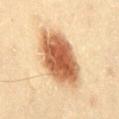Image and clinical context:
A 15 mm close-up extracted from a 3D total-body photography capture. A male patient aged approximately 45. The lesion is located on the abdomen.